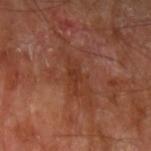Clinical impression:
No biopsy was performed on this lesion — it was imaged during a full skin examination and was not determined to be concerning.
Acquisition and patient details:
The lesion-visualizer software estimated an average lesion color of about L≈35 a*≈24 b*≈30 (CIELAB) and a lesion-to-skin contrast of about 5.5 (normalized; higher = more distinct). It also reported a classifier nevus-likeness of about 0/100. About 5 mm across. The lesion is located on the arm. Imaged with cross-polarized lighting. A male subject, about 70 years old. A 15 mm close-up tile from a total-body photography series done for melanoma screening.